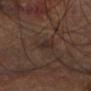Clinical impression:
Recorded during total-body skin imaging; not selected for excision or biopsy.
Context:
A roughly 15 mm field-of-view crop from a total-body skin photograph. A male patient, aged 68–72. The lesion is located on the leg.